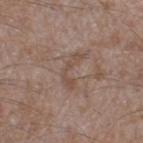Notes:
• subject · male, aged 43 to 47
• lighting · white-light illumination
• body site · the left thigh
• diameter · about 4 mm
• automated metrics · an average lesion color of about L≈48 a*≈16 b*≈25 (CIELAB), about 6 CIELAB-L* units darker than the surrounding skin, and a lesion-to-skin contrast of about 5.5 (normalized; higher = more distinct); a within-lesion color-variation index near 0/10 and a peripheral color-asymmetry measure near 0
• image · ~15 mm crop, total-body skin-cancer survey